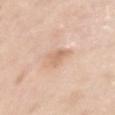{
  "site": "left upper arm",
  "lesion_size": {
    "long_diameter_mm_approx": 3.0
  },
  "patient": {
    "sex": "female",
    "age_approx": 40
  },
  "lighting": "white-light",
  "image": {
    "source": "total-body photography crop",
    "field_of_view_mm": 15
  }
}